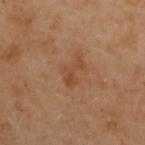Notes:
– biopsy status · no biopsy performed (imaged during a skin exam)
– patient · female, in their 60s
– body site · the upper back
– lighting · cross-polarized illumination
– image · 15 mm crop, total-body photography